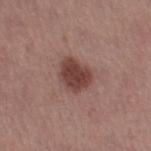biopsy_status: not biopsied; imaged during a skin examination
lighting: white-light
lesion_size:
  long_diameter_mm_approx: 4.0
site: leg
image:
  source: total-body photography crop
  field_of_view_mm: 15
patient:
  sex: female
  age_approx: 50